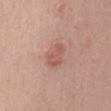{
  "biopsy_status": "not biopsied; imaged during a skin examination",
  "lesion_size": {
    "long_diameter_mm_approx": 3.0
  },
  "patient": {
    "sex": "female",
    "age_approx": 40
  },
  "lighting": "white-light",
  "automated_metrics": {
    "eccentricity": 0.8,
    "shape_asymmetry": 0.25,
    "border_irregularity_0_10": 2.5,
    "color_variation_0_10": 2.5,
    "peripheral_color_asymmetry": 1.0,
    "nevus_likeness_0_100": 35
  },
  "image": {
    "source": "total-body photography crop",
    "field_of_view_mm": 15
  },
  "site": "abdomen"
}